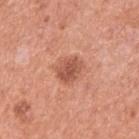follow-up: no biopsy performed (imaged during a skin exam); subject: male, in their mid- to late 50s; image source: ~15 mm tile from a whole-body skin photo; body site: the left upper arm.